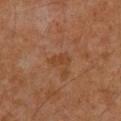Q: Was a biopsy performed?
A: imaged on a skin check; not biopsied
Q: What kind of image is this?
A: 15 mm crop, total-body photography
Q: How was the tile lit?
A: cross-polarized illumination
Q: What is the anatomic site?
A: the mid back
Q: What are the patient's age and sex?
A: male, in their 60s
Q: Lesion size?
A: about 3 mm